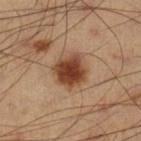biopsy status — no biopsy performed (imaged during a skin exam)
lesion size — ≈4 mm
patient — male, about 40 years old
tile lighting — cross-polarized
imaging modality — ~15 mm tile from a whole-body skin photo
location — the left lower leg